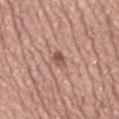Q: Illumination type?
A: white-light
Q: What is the imaging modality?
A: ~15 mm tile from a whole-body skin photo
Q: Patient demographics?
A: male, aged 73 to 77
Q: How large is the lesion?
A: ~2.5 mm (longest diameter)
Q: Lesion location?
A: the front of the torso